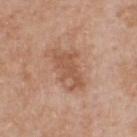Assessment:
Recorded during total-body skin imaging; not selected for excision or biopsy.
Image and clinical context:
A male patient, aged 48–52. A roughly 15 mm field-of-view crop from a total-body skin photograph. Located on the front of the torso.